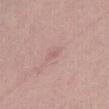The lesion was tiled from a total-body skin photograph and was not biopsied. The subject is a female approximately 40 years of age. Imaged with white-light lighting. A lesion tile, about 15 mm wide, cut from a 3D total-body photograph. On the abdomen.